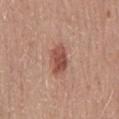Impression: Imaged during a routine full-body skin examination; the lesion was not biopsied and no histopathology is available. Context: Approximately 3.5 mm at its widest. From the mid back. Captured under white-light illumination. A region of skin cropped from a whole-body photographic capture, roughly 15 mm wide. The total-body-photography lesion software estimated a lesion color around L≈51 a*≈24 b*≈27 in CIELAB and a lesion-to-skin contrast of about 8.5 (normalized; higher = more distinct). The software also gave a border-irregularity index near 2.5/10, a color-variation rating of about 4/10, and a peripheral color-asymmetry measure near 1.5. The analysis additionally found a classifier nevus-likeness of about 90/100 and a lesion-detection confidence of about 100/100. A male subject aged 58 to 62.